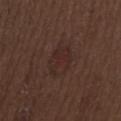Q: Is there a histopathology result?
A: imaged on a skin check; not biopsied